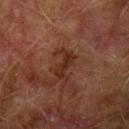– biopsy status · imaged on a skin check; not biopsied
– image source · total-body-photography crop, ~15 mm field of view
– anatomic site · the left upper arm
– lighting · cross-polarized
– size · ~3.5 mm (longest diameter)
– patient · male, roughly 75 years of age
– TBP lesion metrics · a lesion area of about 4.5 mm² and a shape eccentricity near 0.9; a mean CIELAB color near L≈23 a*≈19 b*≈23, a lesion–skin lightness drop of about 7, and a normalized lesion–skin contrast near 8